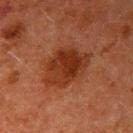Case summary:
– notes · total-body-photography surveillance lesion; no biopsy
– patient · male, aged 58–62
– lesion diameter · about 6 mm
– image source · total-body-photography crop, ~15 mm field of view
– location · the right upper arm